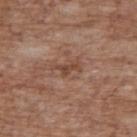Cropped from a total-body skin-imaging series; the visible field is about 15 mm. A male patient, aged approximately 70. The total-body-photography lesion software estimated a footprint of about 2.5 mm², an outline eccentricity of about 0.9 (0 = round, 1 = elongated), and two-axis asymmetry of about 0.55. And it measured an average lesion color of about L≈45 a*≈20 b*≈28 (CIELAB), about 9 CIELAB-L* units darker than the surrounding skin, and a lesion-to-skin contrast of about 7 (normalized; higher = more distinct). The analysis additionally found a border-irregularity index near 6.5/10 and a within-lesion color-variation index near 0/10. The recorded lesion diameter is about 3 mm. The lesion is located on the upper back.